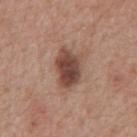No biopsy was performed on this lesion — it was imaged during a full skin examination and was not determined to be concerning.